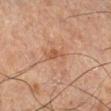{
  "biopsy_status": "not biopsied; imaged during a skin examination",
  "image": {
    "source": "total-body photography crop",
    "field_of_view_mm": 15
  },
  "automated_metrics": {
    "area_mm2_approx": 4.5,
    "shape_asymmetry": 0.3,
    "vs_skin_darker_L": 6.0,
    "vs_skin_contrast_norm": 5.5,
    "nevus_likeness_0_100": 0,
    "lesion_detection_confidence_0_100": 100
  },
  "patient": {
    "sex": "male",
    "age_approx": 65
  },
  "lighting": "cross-polarized",
  "lesion_size": {
    "long_diameter_mm_approx": 3.0
  },
  "site": "right lower leg"
}